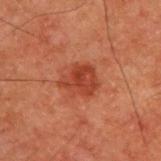This lesion was catalogued during total-body skin photography and was not selected for biopsy.
Automated tile analysis of the lesion measured an average lesion color of about L≈32 a*≈26 b*≈28 (CIELAB), about 8 CIELAB-L* units darker than the surrounding skin, and a normalized border contrast of about 7.5. And it measured a nevus-likeness score of about 50/100 and lesion-presence confidence of about 100/100.
A roughly 15 mm field-of-view crop from a total-body skin photograph.
The lesion's longest dimension is about 4 mm.
Captured under cross-polarized illumination.
Located on the upper back.
A male patient about 50 years old.